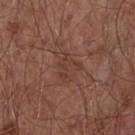This lesion was catalogued during total-body skin photography and was not selected for biopsy.
The lesion-visualizer software estimated an average lesion color of about L≈39 a*≈21 b*≈26 (CIELAB), a lesion–skin lightness drop of about 5, and a lesion-to-skin contrast of about 5 (normalized; higher = more distinct). The software also gave a border-irregularity index near 3.5/10, a within-lesion color-variation index near 3/10, and peripheral color asymmetry of about 1.
A region of skin cropped from a whole-body photographic capture, roughly 15 mm wide.
Approximately 3.5 mm at its widest.
A male subject about 55 years old.
This is a white-light tile.
The lesion is located on the chest.